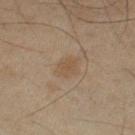| field | value |
|---|---|
| biopsy status | catalogued during a skin exam; not biopsied |
| location | the arm |
| patient | male, in their 50s |
| acquisition | ~15 mm crop, total-body skin-cancer survey |